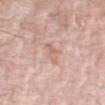workup=imaged on a skin check; not biopsied | imaging modality=~15 mm tile from a whole-body skin photo | lesion diameter=about 2.5 mm | patient=male, aged approximately 75 | illumination=white-light | body site=the arm.